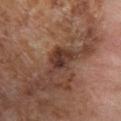Recorded during total-body skin imaging; not selected for excision or biopsy.
The total-body-photography lesion software estimated an area of roughly 9 mm² and an eccentricity of roughly 0.75. And it measured a mean CIELAB color near L≈37 a*≈20 b*≈25 and a lesion-to-skin contrast of about 10 (normalized; higher = more distinct). The software also gave border irregularity of about 4.5 on a 0–10 scale, a color-variation rating of about 5/10, and a peripheral color-asymmetry measure near 1.5. The analysis additionally found a nevus-likeness score of about 10/100 and a lesion-detection confidence of about 95/100.
The lesion is located on the right lower leg.
The tile uses cross-polarized illumination.
Approximately 4.5 mm at its widest.
A 15 mm crop from a total-body photograph taken for skin-cancer surveillance.
A female patient, about 55 years old.